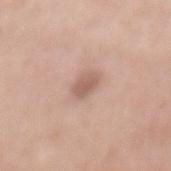Q: Was this lesion biopsied?
A: total-body-photography surveillance lesion; no biopsy
Q: What are the patient's age and sex?
A: female, about 60 years old
Q: Where on the body is the lesion?
A: the mid back
Q: How was this image acquired?
A: ~15 mm tile from a whole-body skin photo
Q: Illumination type?
A: white-light
Q: What is the lesion's diameter?
A: ≈3 mm